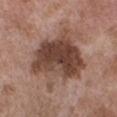Recorded during total-body skin imaging; not selected for excision or biopsy. About 9 mm across. The patient is a male aged around 50. This image is a 15 mm lesion crop taken from a total-body photograph. The lesion is on the chest. The lesion-visualizer software estimated a footprint of about 36 mm², an outline eccentricity of about 0.6 (0 = round, 1 = elongated), and a symmetry-axis asymmetry near 0.3. The software also gave a mean CIELAB color near L≈44 a*≈19 b*≈25, a lesion–skin lightness drop of about 14, and a normalized border contrast of about 10. The software also gave internal color variation of about 7 on a 0–10 scale and a peripheral color-asymmetry measure near 2.5.